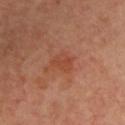| feature | finding |
|---|---|
| imaging modality | ~15 mm crop, total-body skin-cancer survey |
| illumination | cross-polarized |
| subject | male, in their mid-60s |
| anatomic site | the left upper arm |